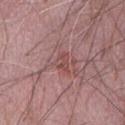Q: Was this lesion biopsied?
A: catalogued during a skin exam; not biopsied